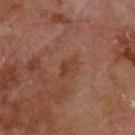Captured during whole-body skin photography for melanoma surveillance; the lesion was not biopsied.
The total-body-photography lesion software estimated a mean CIELAB color near L≈39 a*≈22 b*≈30 and a normalized border contrast of about 6.5. It also reported a border-irregularity rating of about 5/10.
A 15 mm close-up extracted from a 3D total-body photography capture.
On the upper back.
Captured under cross-polarized illumination.
The lesion's longest dimension is about 3 mm.
The subject is a male aged approximately 70.